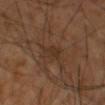This lesion was catalogued during total-body skin photography and was not selected for biopsy.
The recorded lesion diameter is about 3 mm.
The lesion is located on the right arm.
Cropped from a total-body skin-imaging series; the visible field is about 15 mm.
The subject is a male aged 58–62.
The total-body-photography lesion software estimated a border-irregularity rating of about 2.5/10 and a peripheral color-asymmetry measure near 0.5.